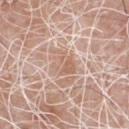  biopsy_status: not biopsied; imaged during a skin examination
  automated_metrics:
    area_mm2_approx: 7.0
    eccentricity: 0.55
    shape_asymmetry: 0.3
    border_irregularity_0_10: 3.5
    color_variation_0_10: 4.0
    peripheral_color_asymmetry: 1.5
    lesion_detection_confidence_0_100: 90
  lesion_size:
    long_diameter_mm_approx: 3.5
  image:
    source: total-body photography crop
    field_of_view_mm: 15
  patient:
    sex: male
    age_approx: 80
  lighting: white-light
  site: chest